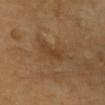Assessment:
The lesion was photographed on a routine skin check and not biopsied; there is no pathology result.
Acquisition and patient details:
The tile uses cross-polarized illumination. The lesion is on the chest. The lesion's longest dimension is about 3 mm. The patient is a male approximately 65 years of age. A roughly 15 mm field-of-view crop from a total-body skin photograph.